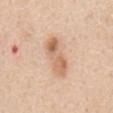Impression: The lesion was photographed on a routine skin check and not biopsied; there is no pathology result. Image and clinical context: A male patient aged 58–62. This is a white-light tile. Measured at roughly 6 mm in maximum diameter. A roughly 15 mm field-of-view crop from a total-body skin photograph. From the mid back.